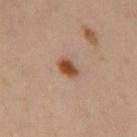Assessment:
This lesion was catalogued during total-body skin photography and was not selected for biopsy.
Context:
Cropped from a whole-body photographic skin survey; the tile spans about 15 mm. A female patient, roughly 40 years of age. From the left thigh. Automated image analysis of the tile measured a lesion area of about 4.5 mm², an outline eccentricity of about 0.75 (0 = round, 1 = elongated), and two-axis asymmetry of about 0.2. The analysis additionally found a mean CIELAB color near L≈49 a*≈22 b*≈32, a lesion–skin lightness drop of about 14, and a lesion-to-skin contrast of about 10.5 (normalized; higher = more distinct). The software also gave a border-irregularity rating of about 2/10, internal color variation of about 5 on a 0–10 scale, and a peripheral color-asymmetry measure near 1.5. It also reported an automated nevus-likeness rating near 100 out of 100 and a lesion-detection confidence of about 100/100. The tile uses cross-polarized illumination.